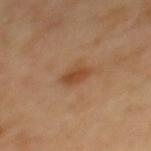This lesion was catalogued during total-body skin photography and was not selected for biopsy. A male patient roughly 65 years of age. This is a cross-polarized tile. This image is a 15 mm lesion crop taken from a total-body photograph. Measured at roughly 2.5 mm in maximum diameter. On the mid back. Automated image analysis of the tile measured a lesion area of about 4 mm², a shape eccentricity near 0.75, and a symmetry-axis asymmetry near 0.25.